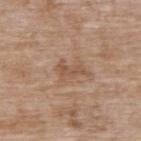Automated image analysis of the tile measured an average lesion color of about L≈53 a*≈18 b*≈30 (CIELAB), a lesion–skin lightness drop of about 8, and a lesion-to-skin contrast of about 5.5 (normalized; higher = more distinct). A 15 mm crop from a total-body photograph taken for skin-cancer surveillance. A female subject aged approximately 75. On the back.